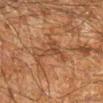The lesion was tiled from a total-body skin photograph and was not biopsied. A male subject about 60 years old. Cropped from a total-body skin-imaging series; the visible field is about 15 mm. The lesion is on the right lower leg.